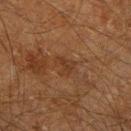Q: Was this lesion biopsied?
A: total-body-photography surveillance lesion; no biopsy
Q: What is the imaging modality?
A: 15 mm crop, total-body photography
Q: Lesion size?
A: ~2.5 mm (longest diameter)
Q: Where on the body is the lesion?
A: the right lower leg
Q: What did automated image analysis measure?
A: an area of roughly 4 mm², an eccentricity of roughly 0.65, and two-axis asymmetry of about 0.4; an average lesion color of about L≈27 a*≈17 b*≈25 (CIELAB), about 5 CIELAB-L* units darker than the surrounding skin, and a lesion-to-skin contrast of about 5.5 (normalized; higher = more distinct); a border-irregularity rating of about 4.5/10 and a color-variation rating of about 1/10
Q: Illumination type?
A: cross-polarized illumination
Q: Who is the patient?
A: male, approximately 60 years of age